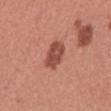follow-up=no biopsy performed (imaged during a skin exam) | lesion diameter=about 3.5 mm | anatomic site=the abdomen | patient=male, in their mid- to late 40s | imaging modality=~15 mm tile from a whole-body skin photo.